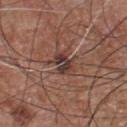A region of skin cropped from a whole-body photographic capture, roughly 15 mm wide. The lesion is on the chest. The subject is a male in their mid-50s.